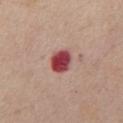| feature | finding |
|---|---|
| notes | no biopsy performed (imaged during a skin exam) |
| lesion diameter | ~3.5 mm (longest diameter) |
| subject | male, approximately 55 years of age |
| imaging modality | ~15 mm tile from a whole-body skin photo |
| lighting | white-light illumination |
| TBP lesion metrics | an average lesion color of about L≈46 a*≈34 b*≈22 (CIELAB) and a lesion–skin lightness drop of about 19; a border-irregularity index near 1.5/10, a within-lesion color-variation index near 4.5/10, and radial color variation of about 1.5; a classifier nevus-likeness of about 0/100 |
| body site | the chest |